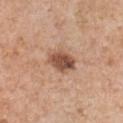Captured during whole-body skin photography for melanoma surveillance; the lesion was not biopsied. A 15 mm close-up tile from a total-body photography series done for melanoma screening. The recorded lesion diameter is about 3.5 mm. Captured under white-light illumination. The lesion is located on the chest. An algorithmic analysis of the crop reported a lesion area of about 7 mm², an eccentricity of roughly 0.65, and a symmetry-axis asymmetry near 0.2. The software also gave an average lesion color of about L≈51 a*≈21 b*≈30 (CIELAB), a lesion–skin lightness drop of about 15, and a normalized border contrast of about 10. The analysis additionally found a border-irregularity rating of about 2/10, a within-lesion color-variation index near 6/10, and peripheral color asymmetry of about 2. The subject is a male aged approximately 60.